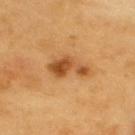biopsy status: imaged on a skin check; not biopsied
automated lesion analysis: a footprint of about 9 mm², an outline eccentricity of about 0.9 (0 = round, 1 = elongated), and two-axis asymmetry of about 0.4; a within-lesion color-variation index near 4/10 and a peripheral color-asymmetry measure near 1.5
acquisition: ~15 mm crop, total-body skin-cancer survey
lighting: cross-polarized illumination
location: the upper back
subject: male, about 60 years old